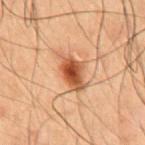follow-up: total-body-photography surveillance lesion; no biopsy
image: ~15 mm crop, total-body skin-cancer survey
tile lighting: cross-polarized
lesion size: ≈4 mm
site: the chest
subject: male, aged 48 to 52